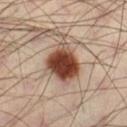{
  "biopsy_status": "not biopsied; imaged during a skin examination",
  "lighting": "cross-polarized",
  "lesion_size": {
    "long_diameter_mm_approx": 4.5
  },
  "site": "right lower leg",
  "image": {
    "source": "total-body photography crop",
    "field_of_view_mm": 15
  },
  "patient": {
    "sex": "male",
    "age_approx": 40
  }
}